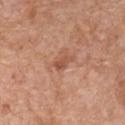workup = no biopsy performed (imaged during a skin exam) | lighting = white-light illumination | anatomic site = the chest | subject = male, aged 58 to 62 | size = ≈2.5 mm | automated lesion analysis = a shape eccentricity near 0.85 and a symmetry-axis asymmetry near 0.35; border irregularity of about 3.5 on a 0–10 scale and a peripheral color-asymmetry measure near 0; lesion-presence confidence of about 100/100 | image = ~15 mm crop, total-body skin-cancer survey.